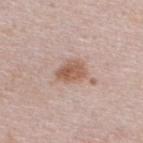The lesion was tiled from a total-body skin photograph and was not biopsied. A male subject, aged around 40. Automated tile analysis of the lesion measured two-axis asymmetry of about 0.2. Imaged with white-light lighting. The lesion is located on the upper back. A roughly 15 mm field-of-view crop from a total-body skin photograph.